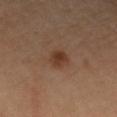follow-up: imaged on a skin check; not biopsied
diameter: ≈2.5 mm
subject: female, approximately 55 years of age
tile lighting: cross-polarized
body site: the right upper arm
TBP lesion metrics: a color-variation rating of about 2.5/10 and radial color variation of about 1; a detector confidence of about 100 out of 100 that the crop contains a lesion
image source: ~15 mm crop, total-body skin-cancer survey